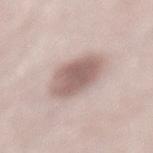This lesion was catalogued during total-body skin photography and was not selected for biopsy.
On the lower back.
A male patient aged 53–57.
Imaged with white-light lighting.
Automated image analysis of the tile measured a lesion–skin lightness drop of about 13 and a lesion-to-skin contrast of about 8.5 (normalized; higher = more distinct).
A 15 mm close-up tile from a total-body photography series done for melanoma screening.